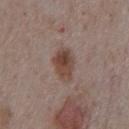Captured during whole-body skin photography for melanoma surveillance; the lesion was not biopsied.
Imaged with white-light lighting.
This image is a 15 mm lesion crop taken from a total-body photograph.
The total-body-photography lesion software estimated an area of roughly 9 mm², an eccentricity of roughly 0.75, and a shape-asymmetry score of about 0.25 (0 = symmetric). And it measured an automated nevus-likeness rating near 95 out of 100 and a lesion-detection confidence of about 100/100.
The patient is a male roughly 75 years of age.
About 4 mm across.
The lesion is on the chest.